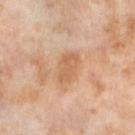Q: Is there a histopathology result?
A: imaged on a skin check; not biopsied
Q: How was the tile lit?
A: cross-polarized
Q: Where on the body is the lesion?
A: the leg
Q: What are the patient's age and sex?
A: female, aged approximately 55
Q: What is the lesion's diameter?
A: about 4 mm
Q: What kind of image is this?
A: 15 mm crop, total-body photography